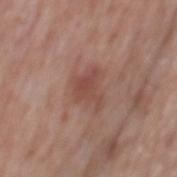Impression:
No biopsy was performed on this lesion — it was imaged during a full skin examination and was not determined to be concerning.
Background:
A male patient, in their mid- to late 60s. Longest diameter approximately 3.5 mm. A region of skin cropped from a whole-body photographic capture, roughly 15 mm wide. Captured under white-light illumination. Automated tile analysis of the lesion measured a footprint of about 5.5 mm². And it measured a nevus-likeness score of about 0/100 and lesion-presence confidence of about 100/100. The lesion is located on the mid back.